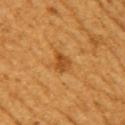A female subject, in their mid- to late 50s.
Cropped from a total-body skin-imaging series; the visible field is about 15 mm.
On the right upper arm.
Automated tile analysis of the lesion measured a mean CIELAB color near L≈50 a*≈27 b*≈47, a lesion–skin lightness drop of about 11, and a normalized lesion–skin contrast near 7.5. And it measured border irregularity of about 3 on a 0–10 scale, internal color variation of about 2.5 on a 0–10 scale, and a peripheral color-asymmetry measure near 1. The software also gave an automated nevus-likeness rating near 65 out of 100 and a lesion-detection confidence of about 100/100.
Imaged with cross-polarized lighting.
The lesion's longest dimension is about 2.5 mm.